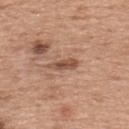Part of a total-body skin-imaging series; this lesion was reviewed on a skin check and was not flagged for biopsy.
On the upper back.
The subject is a female in their mid-50s.
A close-up tile cropped from a whole-body skin photograph, about 15 mm across.